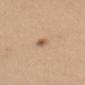Recorded during total-body skin imaging; not selected for excision or biopsy.
A close-up tile cropped from a whole-body skin photograph, about 15 mm across.
An algorithmic analysis of the crop reported about 8 CIELAB-L* units darker than the surrounding skin and a lesion-to-skin contrast of about 5 (normalized; higher = more distinct). The analysis additionally found border irregularity of about 1 on a 0–10 scale, a color-variation rating of about 5.5/10, and radial color variation of about 1.5.
The tile uses white-light illumination.
The lesion is on the chest.
A female patient, in their mid- to late 40s.